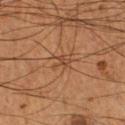workup=catalogued during a skin exam; not biopsied
patient=male, in their mid-50s
diameter=~2.5 mm (longest diameter)
lighting=cross-polarized illumination
body site=the right lower leg
image=total-body-photography crop, ~15 mm field of view